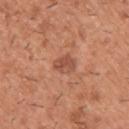No biopsy was performed on this lesion — it was imaged during a full skin examination and was not determined to be concerning.
Approximately 2.5 mm at its widest.
A close-up tile cropped from a whole-body skin photograph, about 15 mm across.
The tile uses white-light illumination.
A male patient aged 38–42.
From the back.